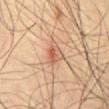Assessment: Captured during whole-body skin photography for melanoma surveillance; the lesion was not biopsied. Context: Captured under cross-polarized illumination. An algorithmic analysis of the crop reported an outline eccentricity of about 0.5 (0 = round, 1 = elongated) and a shape-asymmetry score of about 0.35 (0 = symmetric). The software also gave a lesion color around L≈59 a*≈23 b*≈31 in CIELAB, about 9 CIELAB-L* units darker than the surrounding skin, and a lesion-to-skin contrast of about 6 (normalized; higher = more distinct). The software also gave a within-lesion color-variation index near 7/10 and a peripheral color-asymmetry measure near 2.5. A male subject aged around 55. A region of skin cropped from a whole-body photographic capture, roughly 15 mm wide. About 3 mm across. From the abdomen.